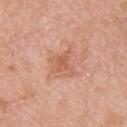Impression:
The lesion was photographed on a routine skin check and not biopsied; there is no pathology result.
Background:
The patient is a male aged 58 to 62. Captured under white-light illumination. Located on the right upper arm. A region of skin cropped from a whole-body photographic capture, roughly 15 mm wide.